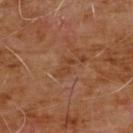Q: Was a biopsy performed?
A: catalogued during a skin exam; not biopsied
Q: What kind of image is this?
A: 15 mm crop, total-body photography
Q: Patient demographics?
A: male, aged around 60
Q: What is the lesion's diameter?
A: ~2.5 mm (longest diameter)
Q: Where on the body is the lesion?
A: the chest
Q: Automated lesion metrics?
A: a shape eccentricity near 0.9 and a shape-asymmetry score of about 0.45 (0 = symmetric); a lesion color around L≈39 a*≈22 b*≈32 in CIELAB and a lesion–skin lightness drop of about 6
Q: What lighting was used for the tile?
A: cross-polarized